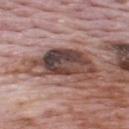The lesion was photographed on a routine skin check and not biopsied; there is no pathology result. Cropped from a whole-body photographic skin survey; the tile spans about 15 mm. The lesion is located on the upper back. Longest diameter approximately 7 mm. The patient is a male roughly 70 years of age.